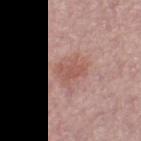biopsy status = no biopsy performed (imaged during a skin exam) | patient = female, roughly 50 years of age | image-analysis metrics = lesion-presence confidence of about 100/100 | acquisition = 15 mm crop, total-body photography | location = the left thigh.